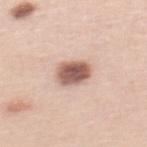Assessment:
Recorded during total-body skin imaging; not selected for excision or biopsy.
Context:
The lesion is located on the mid back. Measured at roughly 3.5 mm in maximum diameter. Captured under white-light illumination. A close-up tile cropped from a whole-body skin photograph, about 15 mm across. The total-body-photography lesion software estimated a shape eccentricity near 0.6 and a shape-asymmetry score of about 0.15 (0 = symmetric). It also reported a mean CIELAB color near L≈58 a*≈20 b*≈25 and a normalized lesion–skin contrast near 11. The software also gave a border-irregularity rating of about 1.5/10, a within-lesion color-variation index near 5/10, and radial color variation of about 1.5. A female patient, aged 43 to 47.